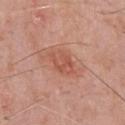Context: A male patient approximately 60 years of age. The lesion is located on the chest. A lesion tile, about 15 mm wide, cut from a 3D total-body photograph. Captured under white-light illumination. Approximately 3.5 mm at its widest.